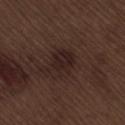Imaged during a routine full-body skin examination; the lesion was not biopsied and no histopathology is available.
A 15 mm close-up tile from a total-body photography series done for melanoma screening.
Imaged with white-light lighting.
The lesion is on the lower back.
A male subject, in their 70s.
The lesion's longest dimension is about 4 mm.